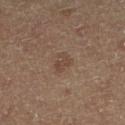biopsy_status: not biopsied; imaged during a skin examination
lighting: cross-polarized
site: right lower leg
patient:
  sex: female
  age_approx: 60
lesion_size:
  long_diameter_mm_approx: 2.5
image:
  source: total-body photography crop
  field_of_view_mm: 15